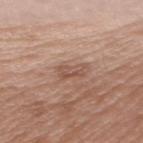{"biopsy_status": "not biopsied; imaged during a skin examination", "image": {"source": "total-body photography crop", "field_of_view_mm": 15}, "site": "left upper arm", "lighting": "white-light", "patient": {"sex": "female", "age_approx": 70}}